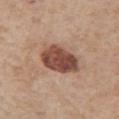{"site": "arm", "lighting": "white-light", "image": {"source": "total-body photography crop", "field_of_view_mm": 15}, "patient": {"sex": "male", "age_approx": 75}}